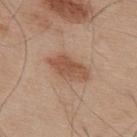No biopsy was performed on this lesion — it was imaged during a full skin examination and was not determined to be concerning. A male patient, aged 53 to 57. The lesion is located on the upper back. Captured under white-light illumination. Longest diameter approximately 5 mm. A close-up tile cropped from a whole-body skin photograph, about 15 mm across.